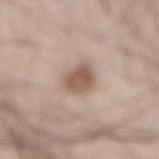| feature | finding |
|---|---|
| biopsy status | total-body-photography surveillance lesion; no biopsy |
| size | ~4 mm (longest diameter) |
| image | 15 mm crop, total-body photography |
| subject | male, approximately 35 years of age |
| anatomic site | the lower back |
| automated metrics | a lesion area of about 8 mm², a shape eccentricity near 0.7, and a symmetry-axis asymmetry near 0.2; border irregularity of about 2 on a 0–10 scale, internal color variation of about 3 on a 0–10 scale, and radial color variation of about 1; a nevus-likeness score of about 95/100 and a lesion-detection confidence of about 100/100 |
| tile lighting | white-light |